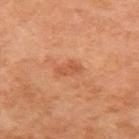Case summary:
* workup — catalogued during a skin exam; not biopsied
* imaging modality — 15 mm crop, total-body photography
* subject — female, approximately 60 years of age
* body site — the right upper arm